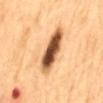The lesion was tiled from a total-body skin photograph and was not biopsied.
A 15 mm close-up tile from a total-body photography series done for melanoma screening.
From the mid back.
A male patient about 65 years old.